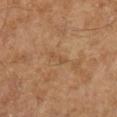workup: no biopsy performed (imaged during a skin exam) | site: the right lower leg | image: ~15 mm crop, total-body skin-cancer survey | TBP lesion metrics: an area of roughly 1.5 mm², an eccentricity of roughly 0.95, and a shape-asymmetry score of about 0.4 (0 = symmetric); a lesion color around L≈45 a*≈17 b*≈32 in CIELAB, roughly 6 lightness units darker than nearby skin, and a normalized lesion–skin contrast near 5; border irregularity of about 5.5 on a 0–10 scale, internal color variation of about 0 on a 0–10 scale, and peripheral color asymmetry of about 0; a nevus-likeness score of about 0/100 and a lesion-detection confidence of about 80/100 | size: ≈2.5 mm | subject: male, roughly 60 years of age | tile lighting: cross-polarized.